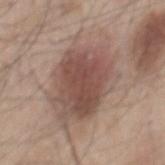Clinical impression:
Part of a total-body skin-imaging series; this lesion was reviewed on a skin check and was not flagged for biopsy.
Image and clinical context:
A male subject, in their mid- to late 40s. An algorithmic analysis of the crop reported a footprint of about 38 mm², an eccentricity of roughly 0.8, and two-axis asymmetry of about 0.25. It also reported a lesion color around L≈51 a*≈18 b*≈23 in CIELAB, about 12 CIELAB-L* units darker than the surrounding skin, and a normalized border contrast of about 8.5. Located on the mid back. A lesion tile, about 15 mm wide, cut from a 3D total-body photograph. About 10 mm across. The tile uses white-light illumination.